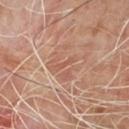<tbp_lesion>
<biopsy_status>not biopsied; imaged during a skin examination</biopsy_status>
<patient>
  <sex>male</sex>
  <age_approx>65</age_approx>
</patient>
<lighting>cross-polarized</lighting>
<site>chest</site>
<automated_metrics>
  <nevus_likeness_0_100>0</nevus_likeness_0_100>
  <lesion_detection_confidence_0_100>80</lesion_detection_confidence_0_100>
</automated_metrics>
<image>
  <source>total-body photography crop</source>
  <field_of_view_mm>15</field_of_view_mm>
</image>
</tbp_lesion>